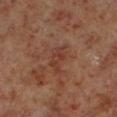Q: Is there a histopathology result?
A: no biopsy performed (imaged during a skin exam)
Q: Patient demographics?
A: male, aged 58–62
Q: What is the imaging modality?
A: 15 mm crop, total-body photography
Q: Automated lesion metrics?
A: a mean CIELAB color near L≈35 a*≈21 b*≈26 and about 6 CIELAB-L* units darker than the surrounding skin; a color-variation rating of about 3.5/10
Q: Lesion location?
A: the leg
Q: How was the tile lit?
A: cross-polarized illumination
Q: Lesion size?
A: ≈4 mm